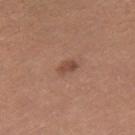Imaged during a routine full-body skin examination; the lesion was not biopsied and no histopathology is available. On the right thigh. A female patient, aged approximately 35. Cropped from a total-body skin-imaging series; the visible field is about 15 mm. This is a white-light tile.